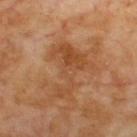Q: Is there a histopathology result?
A: catalogued during a skin exam; not biopsied